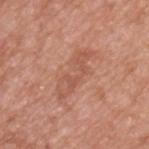| feature | finding |
|---|---|
| image | 15 mm crop, total-body photography |
| lesion diameter | ≈6.5 mm |
| illumination | white-light |
| subject | male, approximately 50 years of age |
| location | the upper back |